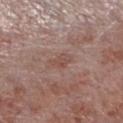biopsy status: catalogued during a skin exam; not biopsied | tile lighting: white-light illumination | body site: the leg | acquisition: ~15 mm crop, total-body skin-cancer survey | patient: male, about 55 years old | automated lesion analysis: a lesion area of about 4 mm², an eccentricity of roughly 0.85, and two-axis asymmetry of about 0.3; a lesion color around L≈49 a*≈20 b*≈23 in CIELAB, about 6 CIELAB-L* units darker than the surrounding skin, and a normalized border contrast of about 5; a border-irregularity index near 3.5/10, a within-lesion color-variation index near 2/10, and radial color variation of about 0.5; a nevus-likeness score of about 0/100 and a lesion-detection confidence of about 100/100 | diameter: about 3 mm.